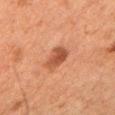Imaged during a routine full-body skin examination; the lesion was not biopsied and no histopathology is available. A female subject roughly 60 years of age. From the arm. A roughly 15 mm field-of-view crop from a total-body skin photograph. About 3 mm across.